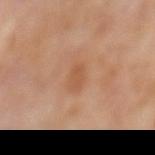| key | value |
|---|---|
| notes | total-body-photography surveillance lesion; no biopsy |